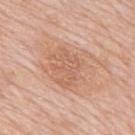This lesion was catalogued during total-body skin photography and was not selected for biopsy.
Cropped from a whole-body photographic skin survey; the tile spans about 15 mm.
The lesion is located on the upper back.
Longest diameter approximately 4 mm.
The total-body-photography lesion software estimated a footprint of about 7.5 mm² and an outline eccentricity of about 0.75 (0 = round, 1 = elongated). And it measured about 7 CIELAB-L* units darker than the surrounding skin and a normalized lesion–skin contrast near 4.5. It also reported a nevus-likeness score of about 0/100 and lesion-presence confidence of about 100/100.
Imaged with white-light lighting.
A male subject, about 80 years old.